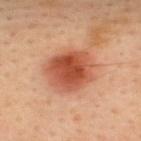Clinical impression: This lesion was catalogued during total-body skin photography and was not selected for biopsy. Image and clinical context: Approximately 5 mm at its widest. A male patient aged around 35. The lesion is on the upper back. Automated tile analysis of the lesion measured a border-irregularity rating of about 1.5/10 and a color-variation rating of about 5.5/10. It also reported a classifier nevus-likeness of about 100/100. Cropped from a total-body skin-imaging series; the visible field is about 15 mm. This is a cross-polarized tile.